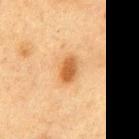Imaged during a routine full-body skin examination; the lesion was not biopsied and no histopathology is available.
The lesion-visualizer software estimated a footprint of about 5.5 mm², an outline eccentricity of about 0.8 (0 = round, 1 = elongated), and a symmetry-axis asymmetry near 0.2.
The tile uses cross-polarized illumination.
A lesion tile, about 15 mm wide, cut from a 3D total-body photograph.
The subject is a male roughly 75 years of age.
On the abdomen.